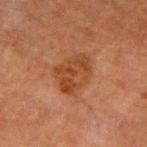Captured during whole-body skin photography for melanoma surveillance; the lesion was not biopsied.
A male subject, about 65 years old.
The total-body-photography lesion software estimated a lesion color around L≈36 a*≈21 b*≈31 in CIELAB, about 7 CIELAB-L* units darker than the surrounding skin, and a normalized lesion–skin contrast near 7.
A region of skin cropped from a whole-body photographic capture, roughly 15 mm wide.
The lesion is located on the left upper arm.
The recorded lesion diameter is about 5 mm.